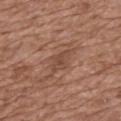follow-up = imaged on a skin check; not biopsied | TBP lesion metrics = a border-irregularity index near 3/10, internal color variation of about 2 on a 0–10 scale, and peripheral color asymmetry of about 0.5; an automated nevus-likeness rating near 0 out of 100 | image source = ~15 mm crop, total-body skin-cancer survey | subject = female, aged approximately 75 | lighting = white-light | lesion size = ≈3 mm | site = the back.